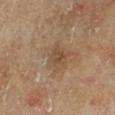The lesion was tiled from a total-body skin photograph and was not biopsied.
On the left lower leg.
A male patient aged around 65.
Automated image analysis of the tile measured a footprint of about 4.5 mm² and two-axis asymmetry of about 0.2. It also reported an average lesion color of about L≈47 a*≈18 b*≈30 (CIELAB), roughly 8 lightness units darker than nearby skin, and a normalized lesion–skin contrast near 6.
The tile uses cross-polarized illumination.
A close-up tile cropped from a whole-body skin photograph, about 15 mm across.